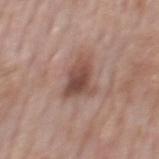Clinical impression: The lesion was tiled from a total-body skin photograph and was not biopsied. Image and clinical context: About 4.5 mm across. The lesion is located on the back. A close-up tile cropped from a whole-body skin photograph, about 15 mm across. The subject is a male about 75 years old. An algorithmic analysis of the crop reported a lesion area of about 10 mm² and a shape eccentricity near 0.75. The analysis additionally found an average lesion color of about L≈49 a*≈20 b*≈24 (CIELAB) and a lesion–skin lightness drop of about 11. The analysis additionally found a peripheral color-asymmetry measure near 1.5. And it measured a lesion-detection confidence of about 100/100. The tile uses white-light illumination.